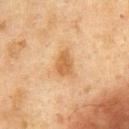The lesion was photographed on a routine skin check and not biopsied; there is no pathology result. The recorded lesion diameter is about 3 mm. From the chest. This is a cross-polarized tile. A 15 mm crop from a total-body photograph taken for skin-cancer surveillance. The subject is a male roughly 75 years of age.